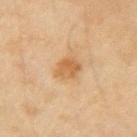Findings:
• body site — the arm
• patient — male, roughly 45 years of age
• imaging modality — ~15 mm tile from a whole-body skin photo